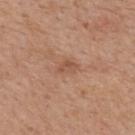Q: Was this lesion biopsied?
A: catalogued during a skin exam; not biopsied
Q: What did automated image analysis measure?
A: an area of roughly 3 mm² and a shape-asymmetry score of about 0.4 (0 = symmetric); border irregularity of about 3.5 on a 0–10 scale and internal color variation of about 1 on a 0–10 scale; a nevus-likeness score of about 0/100 and a detector confidence of about 100 out of 100 that the crop contains a lesion
Q: Who is the patient?
A: male, approximately 65 years of age
Q: Lesion location?
A: the upper back
Q: What lighting was used for the tile?
A: white-light
Q: What is the imaging modality?
A: 15 mm crop, total-body photography
Q: Lesion size?
A: ~2.5 mm (longest diameter)